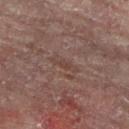Captured during whole-body skin photography for melanoma surveillance; the lesion was not biopsied. The recorded lesion diameter is about 3.5 mm. Automated tile analysis of the lesion measured an area of roughly 3 mm², a shape eccentricity near 0.95, and a symmetry-axis asymmetry near 0.25. And it measured border irregularity of about 3.5 on a 0–10 scale and internal color variation of about 0 on a 0–10 scale. The tile uses cross-polarized illumination. From the leg. A female subject, roughly 80 years of age. A 15 mm crop from a total-body photograph taken for skin-cancer surveillance.